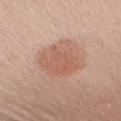The lesion was photographed on a routine skin check and not biopsied; there is no pathology result.
Imaged with white-light lighting.
On the back.
Cropped from a whole-body photographic skin survey; the tile spans about 15 mm.
A female subject aged 48 to 52.
Longest diameter approximately 5.5 mm.